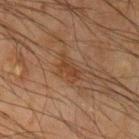Recorded during total-body skin imaging; not selected for excision or biopsy. A region of skin cropped from a whole-body photographic capture, roughly 15 mm wide. A male patient about 65 years old. About 3 mm across. Imaged with cross-polarized lighting. Located on the left upper arm.